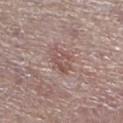  biopsy_status: not biopsied; imaged during a skin examination
  site: left lower leg
  lesion_size:
    long_diameter_mm_approx: 3.0
  image:
    source: total-body photography crop
    field_of_view_mm: 15
  patient:
    sex: female
    age_approx: 65
  lighting: white-light